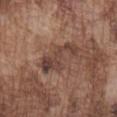Findings:
• notes — catalogued during a skin exam; not biopsied
• size — about 5.5 mm
• patient — male, roughly 75 years of age
• illumination — white-light
• location — the chest
• image — 15 mm crop, total-body photography